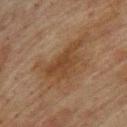Impression:
The lesion was tiled from a total-body skin photograph and was not biopsied.
Context:
About 7 mm across. A close-up tile cropped from a whole-body skin photograph, about 15 mm across. Automated tile analysis of the lesion measured a mean CIELAB color near L≈35 a*≈15 b*≈28 and a normalized lesion–skin contrast near 7. The software also gave border irregularity of about 6.5 on a 0–10 scale, a within-lesion color-variation index near 3/10, and peripheral color asymmetry of about 1. The lesion is on the upper back. The subject is a male about 75 years old.